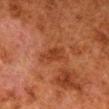Recorded during total-body skin imaging; not selected for excision or biopsy.
Cropped from a whole-body photographic skin survey; the tile spans about 15 mm.
Located on the right lower leg.
The subject is a male in their 80s.